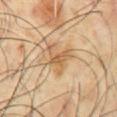The lesion was tiled from a total-body skin photograph and was not biopsied. The subject is a male aged around 60. A roughly 15 mm field-of-view crop from a total-body skin photograph. On the chest. An algorithmic analysis of the crop reported an area of roughly 7 mm². The software also gave an average lesion color of about L≈58 a*≈18 b*≈37 (CIELAB), a lesion–skin lightness drop of about 10, and a lesion-to-skin contrast of about 7 (normalized; higher = more distinct). About 4 mm across.